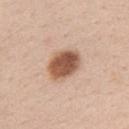Q: Was a biopsy performed?
A: catalogued during a skin exam; not biopsied
Q: Automated lesion metrics?
A: an area of roughly 12 mm², an outline eccentricity of about 0.65 (0 = round, 1 = elongated), and a symmetry-axis asymmetry near 0.1; a border-irregularity index near 1/10 and a peripheral color-asymmetry measure near 1.5; a nevus-likeness score of about 100/100 and a detector confidence of about 100 out of 100 that the crop contains a lesion
Q: How was the tile lit?
A: white-light
Q: How was this image acquired?
A: 15 mm crop, total-body photography
Q: Where on the body is the lesion?
A: the left upper arm
Q: Patient demographics?
A: female, aged 38 to 42